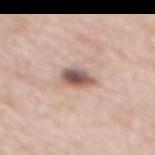notes: imaged on a skin check; not biopsied
image: ~15 mm tile from a whole-body skin photo
subject: female, aged 63 to 67
location: the back